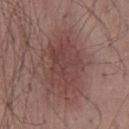Clinical impression:
No biopsy was performed on this lesion — it was imaged during a full skin examination and was not determined to be concerning.
Background:
A male subject aged approximately 50. The lesion is located on the mid back. A close-up tile cropped from a whole-body skin photograph, about 15 mm across.